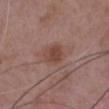Findings:
* biopsy status — no biopsy performed (imaged during a skin exam)
* patient — male, roughly 50 years of age
* tile lighting — white-light illumination
* image-analysis metrics — a lesion area of about 7 mm² and an eccentricity of roughly 0.75; an automated nevus-likeness rating near 55 out of 100 and lesion-presence confidence of about 100/100
* lesion size — ~3.5 mm (longest diameter)
* acquisition — total-body-photography crop, ~15 mm field of view
* location — the chest